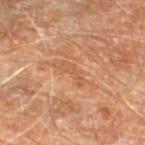Recorded during total-body skin imaging; not selected for excision or biopsy. Located on the left lower leg. The patient is a male about 70 years old. Automated image analysis of the tile measured a lesion color around L≈55 a*≈24 b*≈36 in CIELAB and a lesion–skin lightness drop of about 6. And it measured a border-irregularity rating of about 7.5/10. This image is a 15 mm lesion crop taken from a total-body photograph. Measured at roughly 3 mm in maximum diameter. This is a cross-polarized tile.